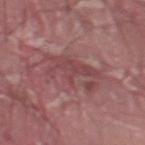Clinical impression:
Recorded during total-body skin imaging; not selected for excision or biopsy.
Image and clinical context:
Measured at roughly 6 mm in maximum diameter. An algorithmic analysis of the crop reported a footprint of about 17 mm², an eccentricity of roughly 0.8, and a symmetry-axis asymmetry near 0.4. And it measured a lesion color around L≈46 a*≈25 b*≈19 in CIELAB and a lesion–skin lightness drop of about 7. The analysis additionally found a within-lesion color-variation index near 3/10 and radial color variation of about 1. The analysis additionally found a classifier nevus-likeness of about 0/100. The tile uses white-light illumination. On the left lower leg. A male subject aged around 40. A 15 mm close-up tile from a total-body photography series done for melanoma screening.